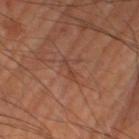follow-up = imaged on a skin check; not biopsied
subject = male, aged around 70
site = the right thigh
acquisition = 15 mm crop, total-body photography